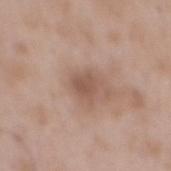{
  "biopsy_status": "not biopsied; imaged during a skin examination",
  "patient": {
    "sex": "male",
    "age_approx": 50
  },
  "image": {
    "source": "total-body photography crop",
    "field_of_view_mm": 15
  },
  "lighting": "white-light",
  "site": "mid back",
  "lesion_size": {
    "long_diameter_mm_approx": 3.0
  }
}